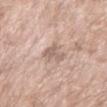| key | value |
|---|---|
| workup | imaged on a skin check; not biopsied |
| lesion diameter | about 3 mm |
| tile lighting | white-light |
| image source | ~15 mm tile from a whole-body skin photo |
| site | the left forearm |
| image-analysis metrics | an outline eccentricity of about 0.8 (0 = round, 1 = elongated); an average lesion color of about L≈61 a*≈16 b*≈25 (CIELAB), a lesion–skin lightness drop of about 9, and a normalized lesion–skin contrast near 6; a border-irregularity index near 4.5/10 and a color-variation rating of about 1/10; a nevus-likeness score of about 0/100 and lesion-presence confidence of about 95/100 |
| subject | female, in their mid- to late 70s |